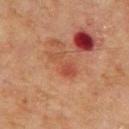workup — imaged on a skin check; not biopsied
illumination — cross-polarized
imaging modality — 15 mm crop, total-body photography
TBP lesion metrics — a mean CIELAB color near L≈40 a*≈24 b*≈29 and a lesion-to-skin contrast of about 5.5 (normalized; higher = more distinct)
subject — male, about 70 years old
body site — the mid back
size — ~4 mm (longest diameter)